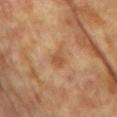biopsy status = imaged on a skin check; not biopsied
image-analysis metrics = a footprint of about 4 mm², an eccentricity of roughly 0.65, and two-axis asymmetry of about 0.3; about 8 CIELAB-L* units darker than the surrounding skin and a normalized border contrast of about 6; a within-lesion color-variation index near 3/10 and peripheral color asymmetry of about 1; a detector confidence of about 100 out of 100 that the crop contains a lesion
imaging modality = total-body-photography crop, ~15 mm field of view
patient = female, in their mid- to late 70s
body site = the upper back
lighting = cross-polarized illumination
lesion size = ~2.5 mm (longest diameter)